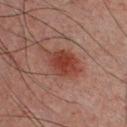Q: Where on the body is the lesion?
A: the chest
Q: What is the imaging modality?
A: ~15 mm tile from a whole-body skin photo
Q: How was the tile lit?
A: cross-polarized
Q: What is the lesion's diameter?
A: ~4 mm (longest diameter)
Q: Who is the patient?
A: male, about 30 years old
Q: Automated lesion metrics?
A: an area of roughly 10 mm², a shape eccentricity near 0.7, and two-axis asymmetry of about 0.15; a classifier nevus-likeness of about 100/100 and lesion-presence confidence of about 100/100